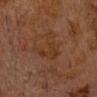Imaged during a routine full-body skin examination; the lesion was not biopsied and no histopathology is available. The lesion-visualizer software estimated a lesion color around L≈28 a*≈17 b*≈26 in CIELAB and a lesion-to-skin contrast of about 5.5 (normalized; higher = more distinct). It also reported border irregularity of about 9.5 on a 0–10 scale, a within-lesion color-variation index near 1.5/10, and radial color variation of about 0.5. It also reported a classifier nevus-likeness of about 0/100 and a lesion-detection confidence of about 95/100. A 15 mm crop from a total-body photograph taken for skin-cancer surveillance. A male subject aged 73–77. The lesion is on the head or neck. About 5.5 mm across.